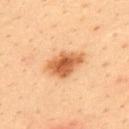Impression:
Recorded during total-body skin imaging; not selected for excision or biopsy.
Image and clinical context:
Automated tile analysis of the lesion measured an area of roughly 11 mm², a shape eccentricity near 0.75, and a shape-asymmetry score of about 0.2 (0 = symmetric). And it measured a border-irregularity index near 2/10, internal color variation of about 5 on a 0–10 scale, and radial color variation of about 1.5. The software also gave a nevus-likeness score of about 95/100 and a lesion-detection confidence of about 100/100. A male patient aged 33 to 37. The tile uses cross-polarized illumination. A 15 mm close-up tile from a total-body photography series done for melanoma screening. The lesion's longest dimension is about 5 mm. Located on the upper back.